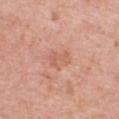Clinical impression: The lesion was tiled from a total-body skin photograph and was not biopsied. Clinical summary: A roughly 15 mm field-of-view crop from a total-body skin photograph. Measured at roughly 3 mm in maximum diameter. Imaged with white-light lighting. An algorithmic analysis of the crop reported a footprint of about 5.5 mm², an outline eccentricity of about 0.7 (0 = round, 1 = elongated), and a symmetry-axis asymmetry near 0.35. From the chest. A female patient, in their 40s.